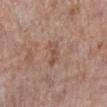biopsy status: total-body-photography surveillance lesion; no biopsy
lighting: white-light illumination
location: the right lower leg
imaging modality: ~15 mm tile from a whole-body skin photo
size: about 3 mm
patient: female, roughly 70 years of age